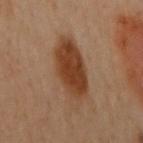Part of a total-body skin-imaging series; this lesion was reviewed on a skin check and was not flagged for biopsy. A female subject aged 58 to 62. The total-body-photography lesion software estimated an average lesion color of about L≈35 a*≈18 b*≈29 (CIELAB), about 10 CIELAB-L* units darker than the surrounding skin, and a normalized lesion–skin contrast near 10. It also reported a border-irregularity rating of about 2.5/10, internal color variation of about 4 on a 0–10 scale, and peripheral color asymmetry of about 1.5. The lesion is located on the left arm. Measured at roughly 7 mm in maximum diameter. Captured under cross-polarized illumination. A 15 mm crop from a total-body photograph taken for skin-cancer surveillance.